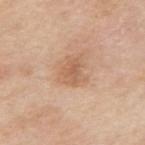Part of a total-body skin-imaging series; this lesion was reviewed on a skin check and was not flagged for biopsy.
The lesion is located on the right upper arm.
A male patient, aged approximately 80.
This is a white-light tile.
The recorded lesion diameter is about 3.5 mm.
Cropped from a whole-body photographic skin survey; the tile spans about 15 mm.